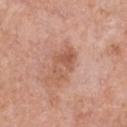Assessment: The lesion was tiled from a total-body skin photograph and was not biopsied. Context: Cropped from a total-body skin-imaging series; the visible field is about 15 mm. Automated tile analysis of the lesion measured a lesion area of about 7 mm² and a shape eccentricity near 0.85. It also reported a mean CIELAB color near L≈56 a*≈24 b*≈31, a lesion–skin lightness drop of about 9, and a normalized border contrast of about 6.5. The software also gave border irregularity of about 5.5 on a 0–10 scale, a within-lesion color-variation index near 3/10, and radial color variation of about 1. The analysis additionally found an automated nevus-likeness rating near 0 out of 100 and a detector confidence of about 100 out of 100 that the crop contains a lesion. Located on the chest. The recorded lesion diameter is about 4 mm. The tile uses white-light illumination. A male patient in their 70s.